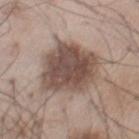| key | value |
|---|---|
| image source | total-body-photography crop, ~15 mm field of view |
| diameter | about 7.5 mm |
| anatomic site | the chest |
| lighting | white-light |
| subject | male, aged 53–57 |